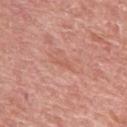<case>
  <biopsy_status>not biopsied; imaged during a skin examination</biopsy_status>
  <lesion_size>
    <long_diameter_mm_approx>2.5</long_diameter_mm_approx>
  </lesion_size>
  <image>
    <source>total-body photography crop</source>
    <field_of_view_mm>15</field_of_view_mm>
  </image>
  <site>left upper arm</site>
  <lighting>white-light</lighting>
  <patient>
    <sex>female</sex>
    <age_approx>60</age_approx>
  </patient>
</case>